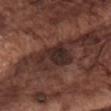workup = catalogued during a skin exam; not biopsied | subject = male, about 75 years old | body site = the chest | imaging modality = 15 mm crop, total-body photography | image-analysis metrics = a border-irregularity rating of about 5/10.